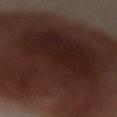Case summary:
• workup: imaged on a skin check; not biopsied